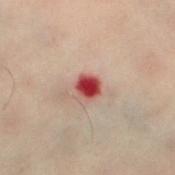site — the leg; patient — female, aged approximately 60; image source — total-body-photography crop, ~15 mm field of view.